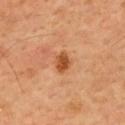Clinical impression:
Part of a total-body skin-imaging series; this lesion was reviewed on a skin check and was not flagged for biopsy.
Image and clinical context:
The tile uses cross-polarized illumination. A male subject, approximately 55 years of age. From the upper back. The lesion-visualizer software estimated a lesion area of about 4 mm², an eccentricity of roughly 0.6, and a symmetry-axis asymmetry near 0.2. It also reported a lesion color around L≈51 a*≈27 b*≈41 in CIELAB, roughly 13 lightness units darker than nearby skin, and a normalized border contrast of about 9. It also reported a within-lesion color-variation index near 2.5/10 and peripheral color asymmetry of about 1. A close-up tile cropped from a whole-body skin photograph, about 15 mm across. Longest diameter approximately 2.5 mm.